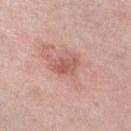Clinical summary:
This image is a 15 mm lesion crop taken from a total-body photograph. From the right lower leg. Imaged with white-light lighting. A female subject aged approximately 40.